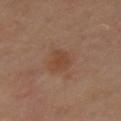| key | value |
|---|---|
| workup | total-body-photography surveillance lesion; no biopsy |
| anatomic site | the left upper arm |
| automated lesion analysis | a footprint of about 5.5 mm², an eccentricity of roughly 0.7, and two-axis asymmetry of about 0.35; a border-irregularity index near 3.5/10, internal color variation of about 2 on a 0–10 scale, and a peripheral color-asymmetry measure near 0.5; a nevus-likeness score of about 60/100 and a detector confidence of about 100 out of 100 that the crop contains a lesion |
| size | ≈3 mm |
| imaging modality | 15 mm crop, total-body photography |